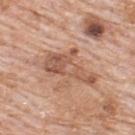biopsy_status: not biopsied; imaged during a skin examination
lighting: white-light
image:
  source: total-body photography crop
  field_of_view_mm: 15
patient:
  sex: male
  age_approx: 80
site: upper back